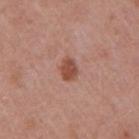Case summary:
* workup: imaged on a skin check; not biopsied
* image source: 15 mm crop, total-body photography
* illumination: white-light illumination
* image-analysis metrics: a lesion area of about 4.5 mm² and a symmetry-axis asymmetry near 0.3; a border-irregularity rating of about 2.5/10, internal color variation of about 2 on a 0–10 scale, and peripheral color asymmetry of about 0.5; an automated nevus-likeness rating near 95 out of 100 and a lesion-detection confidence of about 100/100
* lesion size: ~2.5 mm (longest diameter)
* patient: female, aged 38 to 42
* anatomic site: the right upper arm